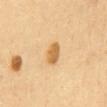Part of a total-body skin-imaging series; this lesion was reviewed on a skin check and was not flagged for biopsy. Cropped from a total-body skin-imaging series; the visible field is about 15 mm. Located on the front of the torso. Captured under cross-polarized illumination. The lesion's longest dimension is about 2.5 mm. A female patient, in their 50s.